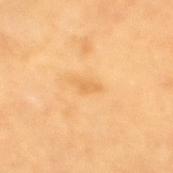<record>
  <biopsy_status>not biopsied; imaged during a skin examination</biopsy_status>
  <patient>
    <sex>female</sex>
    <age_approx>70</age_approx>
  </patient>
  <site>back</site>
  <image>
    <source>total-body photography crop</source>
    <field_of_view_mm>15</field_of_view_mm>
  </image>
  <automated_metrics>
    <area_mm2_approx>2.5</area_mm2_approx>
    <eccentricity>0.9</eccentricity>
    <shape_asymmetry>0.4</shape_asymmetry>
    <vs_skin_contrast_norm>5.0</vs_skin_contrast_norm>
    <nevus_likeness_0_100>0</nevus_likeness_0_100>
    <lesion_detection_confidence_0_100>100</lesion_detection_confidence_0_100>
  </automated_metrics>
</record>